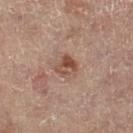No biopsy was performed on this lesion — it was imaged during a full skin examination and was not determined to be concerning.
Longest diameter approximately 2.5 mm.
A close-up tile cropped from a whole-body skin photograph, about 15 mm across.
A female subject, approximately 80 years of age.
The lesion is on the right leg.
Imaged with cross-polarized lighting.